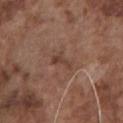Q: Was a biopsy performed?
A: imaged on a skin check; not biopsied
Q: What lighting was used for the tile?
A: white-light illumination
Q: Automated lesion metrics?
A: a footprint of about 2.5 mm², an outline eccentricity of about 0.9 (0 = round, 1 = elongated), and a shape-asymmetry score of about 0.55 (0 = symmetric); roughly 7 lightness units darker than nearby skin and a normalized border contrast of about 6.5; a border-irregularity index near 5.5/10; an automated nevus-likeness rating near 0 out of 100
Q: What kind of image is this?
A: ~15 mm tile from a whole-body skin photo
Q: What is the anatomic site?
A: the chest
Q: How large is the lesion?
A: ~2.5 mm (longest diameter)
Q: Who is the patient?
A: male, aged approximately 75